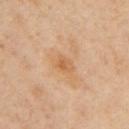workup — no biopsy performed (imaged during a skin exam)
image-analysis metrics — an automated nevus-likeness rating near 0 out of 100
patient — female, aged around 40
size — ≈2.5 mm
image — ~15 mm tile from a whole-body skin photo
site — the left arm
tile lighting — cross-polarized illumination